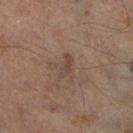Imaged during a routine full-body skin examination; the lesion was not biopsied and no histopathology is available. A 15 mm close-up tile from a total-body photography series done for melanoma screening. The lesion is located on the right lower leg. The patient is a male about 65 years old.